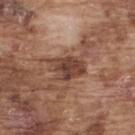Background: Cropped from a total-body skin-imaging series; the visible field is about 15 mm. Measured at roughly 4 mm in maximum diameter. The patient is a male approximately 75 years of age. Imaged with white-light lighting. From the upper back.